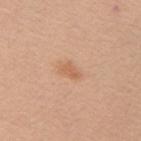biopsy status = imaged on a skin check; not biopsied.